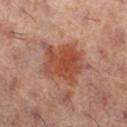biopsy status = no biopsy performed (imaged during a skin exam); acquisition = 15 mm crop, total-body photography; size = ~6.5 mm (longest diameter); site = the leg; lighting = cross-polarized illumination; patient = female, aged around 60.